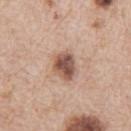follow-up: catalogued during a skin exam; not biopsied | lighting: white-light illumination | subject: male, aged 63 to 67 | automated metrics: a lesion color around L≈54 a*≈20 b*≈27 in CIELAB, a lesion–skin lightness drop of about 15, and a lesion-to-skin contrast of about 9.5 (normalized; higher = more distinct) | location: the right upper arm | image source: ~15 mm crop, total-body skin-cancer survey | lesion size: about 3.5 mm.